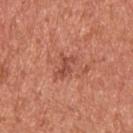biopsy status = total-body-photography surveillance lesion; no biopsy
automated metrics = about 9 CIELAB-L* units darker than the surrounding skin and a normalized lesion–skin contrast near 6.5; a border-irregularity rating of about 5/10, a color-variation rating of about 0/10, and a peripheral color-asymmetry measure near 0
lesion size = ≈3 mm
location = the upper back
image source = total-body-photography crop, ~15 mm field of view
subject = male, in their mid-50s